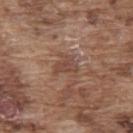Clinical impression: Part of a total-body skin-imaging series; this lesion was reviewed on a skin check and was not flagged for biopsy. Background: A male subject, aged 73 to 77. Imaged with white-light lighting. The total-body-photography lesion software estimated a lesion color around L≈45 a*≈19 b*≈26 in CIELAB, a lesion–skin lightness drop of about 8, and a lesion-to-skin contrast of about 6 (normalized; higher = more distinct). Located on the upper back. Measured at roughly 3 mm in maximum diameter. A 15 mm crop from a total-body photograph taken for skin-cancer surveillance.